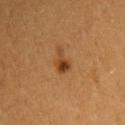<case>
  <image>
    <source>total-body photography crop</source>
    <field_of_view_mm>15</field_of_view_mm>
  </image>
  <lesion_size>
    <long_diameter_mm_approx>3.0</long_diameter_mm_approx>
  </lesion_size>
  <lighting>cross-polarized</lighting>
  <site>left arm</site>
  <patient>
    <sex>female</sex>
    <age_approx>30</age_approx>
  </patient>
</case>